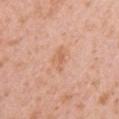The lesion is located on the right upper arm.
Cropped from a total-body skin-imaging series; the visible field is about 15 mm.
A female patient about 40 years old.
The tile uses white-light illumination.
Automated image analysis of the tile measured a lesion color around L≈63 a*≈23 b*≈35 in CIELAB and about 7 CIELAB-L* units darker than the surrounding skin. It also reported a border-irregularity index near 4/10 and a peripheral color-asymmetry measure near 0.5.